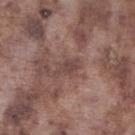  biopsy_status: not biopsied; imaged during a skin examination